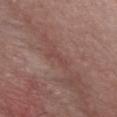Case summary:
• body site · the chest
• automated metrics · an average lesion color of about L≈45 a*≈22 b*≈22 (CIELAB) and about 5 CIELAB-L* units darker than the surrounding skin; a border-irregularity index near 6/10 and a color-variation rating of about 0/10
• patient · male, roughly 45 years of age
• lesion diameter · ~2.5 mm (longest diameter)
• image · 15 mm crop, total-body photography
• lighting · white-light